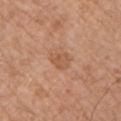The lesion was photographed on a routine skin check and not biopsied; there is no pathology result. A male subject, aged 63 to 67. Captured under white-light illumination. This image is a 15 mm lesion crop taken from a total-body photograph. The lesion's longest dimension is about 2.5 mm. From the arm.